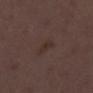Q: Was this lesion biopsied?
A: imaged on a skin check; not biopsied
Q: What kind of image is this?
A: total-body-photography crop, ~15 mm field of view
Q: Where on the body is the lesion?
A: the left lower leg
Q: Patient demographics?
A: female, aged approximately 50
Q: What did automated image analysis measure?
A: an area of roughly 3 mm², an eccentricity of roughly 0.85, and a symmetry-axis asymmetry near 0.45
Q: How large is the lesion?
A: ≈3 mm
Q: What lighting was used for the tile?
A: white-light illumination